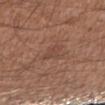The lesion was photographed on a routine skin check and not biopsied; there is no pathology result. About 3 mm across. The patient is a male about 50 years old. A 15 mm crop from a total-body photograph taken for skin-cancer surveillance. The lesion is located on the arm.